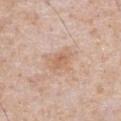Notes:
– follow-up · imaged on a skin check; not biopsied
– image-analysis metrics · a mean CIELAB color near L≈62 a*≈20 b*≈32, about 7 CIELAB-L* units darker than the surrounding skin, and a normalized border contrast of about 6; a nevus-likeness score of about 0/100 and a detector confidence of about 100 out of 100 that the crop contains a lesion
– site · the abdomen
– tile lighting · white-light illumination
– patient · male, in their mid- to late 60s
– acquisition · ~15 mm tile from a whole-body skin photo
– lesion diameter · about 2.5 mm